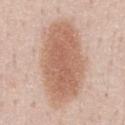Notes:
• anatomic site — the abdomen
• tile lighting — white-light illumination
• lesion size — ≈10.5 mm
• patient — male, roughly 60 years of age
• acquisition — ~15 mm crop, total-body skin-cancer survey